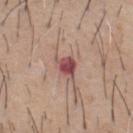| key | value |
|---|---|
| workup | imaged on a skin check; not biopsied |
| image source | 15 mm crop, total-body photography |
| diameter | ≈3 mm |
| body site | the chest |
| illumination | white-light |
| subject | male, aged 58–62 |